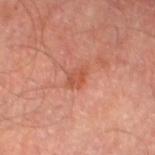Part of a total-body skin-imaging series; this lesion was reviewed on a skin check and was not flagged for biopsy. This is a cross-polarized tile. Longest diameter approximately 2.5 mm. A 15 mm close-up tile from a total-body photography series done for melanoma screening. A male subject about 70 years old. Located on the left thigh.